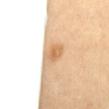Impression:
This lesion was catalogued during total-body skin photography and was not selected for biopsy.
Image and clinical context:
Captured under cross-polarized illumination. A 15 mm close-up extracted from a 3D total-body photography capture. A male subject, aged 63–67. Measured at roughly 3.5 mm in maximum diameter. The lesion is on the back. Automated image analysis of the tile measured a mean CIELAB color near L≈69 a*≈18 b*≈38, a lesion–skin lightness drop of about 12, and a lesion-to-skin contrast of about 8.5 (normalized; higher = more distinct). And it measured radial color variation of about 1.5.